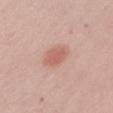This lesion was catalogued during total-body skin photography and was not selected for biopsy.
Located on the left upper arm.
A male subject, approximately 50 years of age.
Longest diameter approximately 3.5 mm.
A close-up tile cropped from a whole-body skin photograph, about 15 mm across.
The total-body-photography lesion software estimated a border-irregularity rating of about 2/10, a within-lesion color-variation index near 2/10, and radial color variation of about 0.5.
Imaged with white-light lighting.